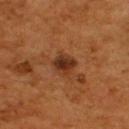Assessment: Part of a total-body skin-imaging series; this lesion was reviewed on a skin check and was not flagged for biopsy. Image and clinical context: The lesion is on the back. The tile uses cross-polarized illumination. Measured at roughly 2.5 mm in maximum diameter. A male subject about 55 years old. Cropped from a whole-body photographic skin survey; the tile spans about 15 mm. Automated image analysis of the tile measured a within-lesion color-variation index near 4.5/10 and a peripheral color-asymmetry measure near 1.5.